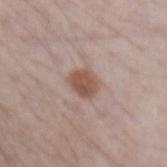Impression:
This lesion was catalogued during total-body skin photography and was not selected for biopsy.
Image and clinical context:
A male patient roughly 70 years of age. Approximately 3 mm at its widest. The lesion is located on the left forearm. Automated image analysis of the tile measured a mean CIELAB color near L≈52 a*≈19 b*≈25, about 11 CIELAB-L* units darker than the surrounding skin, and a normalized lesion–skin contrast near 8.5. And it measured a border-irregularity index near 2/10, internal color variation of about 2.5 on a 0–10 scale, and radial color variation of about 1. Cropped from a total-body skin-imaging series; the visible field is about 15 mm. Captured under white-light illumination.